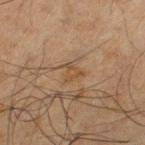Part of a total-body skin-imaging series; this lesion was reviewed on a skin check and was not flagged for biopsy. The lesion is on the left thigh. A male patient, aged 63–67. A 15 mm close-up tile from a total-body photography series done for melanoma screening.